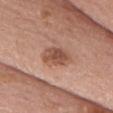Captured during whole-body skin photography for melanoma surveillance; the lesion was not biopsied. A lesion tile, about 15 mm wide, cut from a 3D total-body photograph. Captured under white-light illumination. Approximately 3.5 mm at its widest. A female patient roughly 60 years of age. An algorithmic analysis of the crop reported a lesion area of about 7 mm², an eccentricity of roughly 0.75, and a symmetry-axis asymmetry near 0.2. Located on the head or neck.